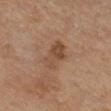Impression:
This lesion was catalogued during total-body skin photography and was not selected for biopsy.
Clinical summary:
A female patient about 55 years old. About 4 mm across. Captured under cross-polarized illumination. This image is a 15 mm lesion crop taken from a total-body photograph. On the right lower leg. An algorithmic analysis of the crop reported a footprint of about 8 mm², an outline eccentricity of about 0.85 (0 = round, 1 = elongated), and a symmetry-axis asymmetry near 0.3. The software also gave a nevus-likeness score of about 0/100 and a detector confidence of about 100 out of 100 that the crop contains a lesion.